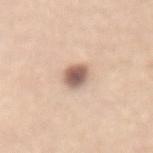Findings:
– notes: imaged on a skin check; not biopsied
– imaging modality: ~15 mm crop, total-body skin-cancer survey
– diameter: ≈3 mm
– site: the back
– patient: female, aged 63 to 67